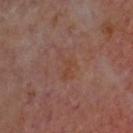workup = total-body-photography surveillance lesion; no biopsy, patient = approximately 65 years of age, anatomic site = the head or neck, lighting = cross-polarized illumination, acquisition = ~15 mm tile from a whole-body skin photo.